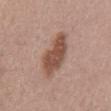<lesion>
<biopsy_status>not biopsied; imaged during a skin examination</biopsy_status>
<image>
  <source>total-body photography crop</source>
  <field_of_view_mm>15</field_of_view_mm>
</image>
<patient>
  <sex>male</sex>
  <age_approx>55</age_approx>
</patient>
<lighting>white-light</lighting>
<lesion_size>
  <long_diameter_mm_approx>6.0</long_diameter_mm_approx>
</lesion_size>
</lesion>